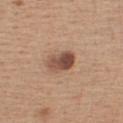Findings:
• biopsy status: catalogued during a skin exam; not biopsied
• diameter: about 4 mm
• patient: female, aged around 45
• location: the front of the torso
• acquisition: total-body-photography crop, ~15 mm field of view